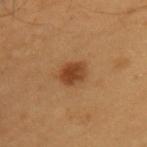Clinical impression: Recorded during total-body skin imaging; not selected for excision or biopsy. Acquisition and patient details: Measured at roughly 3 mm in maximum diameter. A lesion tile, about 15 mm wide, cut from a 3D total-body photograph. The patient is a male approximately 55 years of age. The lesion-visualizer software estimated an area of roughly 6 mm² and a shape-asymmetry score of about 0.15 (0 = symmetric). It also reported a lesion–skin lightness drop of about 11 and a lesion-to-skin contrast of about 9 (normalized; higher = more distinct). And it measured a color-variation rating of about 3/10. Located on the back. Imaged with cross-polarized lighting.